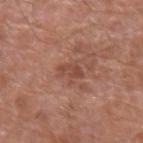{"biopsy_status": "not biopsied; imaged during a skin examination", "image": {"source": "total-body photography crop", "field_of_view_mm": 15}, "patient": {"sex": "male", "age_approx": 80}, "lesion_size": {"long_diameter_mm_approx": 3.0}, "automated_metrics": {"vs_skin_darker_L": 8.0, "vs_skin_contrast_norm": 6.0, "border_irregularity_0_10": 4.0, "color_variation_0_10": 3.0, "peripheral_color_asymmetry": 1.0}, "site": "right forearm"}